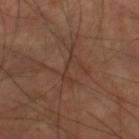Assessment: Imaged during a routine full-body skin examination; the lesion was not biopsied and no histopathology is available. Image and clinical context: A close-up tile cropped from a whole-body skin photograph, about 15 mm across. From the right lower leg. The tile uses cross-polarized illumination. Longest diameter approximately 4.5 mm. The subject is a male approximately 60 years of age.